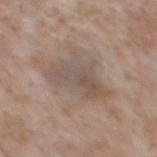| field | value |
|---|---|
| follow-up | total-body-photography surveillance lesion; no biopsy |